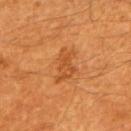Imaged during a routine full-body skin examination; the lesion was not biopsied and no histopathology is available.
A male patient in their 60s.
The lesion's longest dimension is about 4 mm.
The lesion is on the mid back.
A 15 mm close-up tile from a total-body photography series done for melanoma screening.